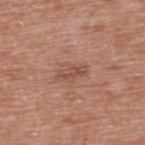workup — no biopsy performed (imaged during a skin exam)
image — ~15 mm tile from a whole-body skin photo
lighting — white-light
site — the upper back
image-analysis metrics — an average lesion color of about L≈50 a*≈23 b*≈28 (CIELAB) and a lesion–skin lightness drop of about 8; a border-irregularity index near 3.5/10, internal color variation of about 0 on a 0–10 scale, and peripheral color asymmetry of about 0
subject — male, aged 63 to 67